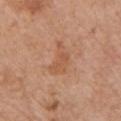| field | value |
|---|---|
| biopsy status | no biopsy performed (imaged during a skin exam) |
| subject | male, in their 80s |
| image-analysis metrics | an area of roughly 6 mm², an eccentricity of roughly 0.85, and a symmetry-axis asymmetry near 0.5; a classifier nevus-likeness of about 0/100 and lesion-presence confidence of about 100/100 |
| illumination | white-light illumination |
| acquisition | ~15 mm tile from a whole-body skin photo |
| body site | the chest |
| lesion size | about 4.5 mm |